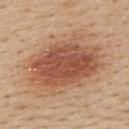<case>
<biopsy_status>not biopsied; imaged during a skin examination</biopsy_status>
<patient>
  <sex>female</sex>
  <age_approx>45</age_approx>
</patient>
<site>upper back</site>
<image>
  <source>total-body photography crop</source>
  <field_of_view_mm>15</field_of_view_mm>
</image>
<lighting>white-light</lighting>
<automated_metrics>
  <area_mm2_approx>42.0</area_mm2_approx>
  <shape_asymmetry>0.15</shape_asymmetry>
  <nevus_likeness_0_100>100</nevus_likeness_0_100>
</automated_metrics>
<lesion_size>
  <long_diameter_mm_approx>9.0</long_diameter_mm_approx>
</lesion_size>
</case>